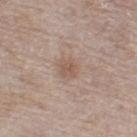| feature | finding |
|---|---|
| biopsy status | total-body-photography surveillance lesion; no biopsy |
| image | ~15 mm crop, total-body skin-cancer survey |
| subject | female, about 60 years old |
| location | the left thigh |